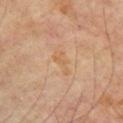Part of a total-body skin-imaging series; this lesion was reviewed on a skin check and was not flagged for biopsy.
Cropped from a total-body skin-imaging series; the visible field is about 15 mm.
Located on the front of the torso.
Imaged with cross-polarized lighting.
Automated image analysis of the tile measured a lesion area of about 3.5 mm². The software also gave an average lesion color of about L≈60 a*≈19 b*≈37 (CIELAB), a lesion–skin lightness drop of about 5, and a lesion-to-skin contrast of about 5 (normalized; higher = more distinct).
A male patient aged around 70.